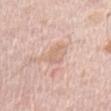Part of a total-body skin-imaging series; this lesion was reviewed on a skin check and was not flagged for biopsy. The lesion is on the chest. The subject is a male aged 68 to 72. Captured under white-light illumination. An algorithmic analysis of the crop reported an average lesion color of about L≈67 a*≈19 b*≈29 (CIELAB) and roughly 7 lightness units darker than nearby skin. A roughly 15 mm field-of-view crop from a total-body skin photograph. The recorded lesion diameter is about 2.5 mm.